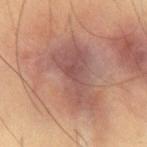{
  "biopsy_status": "not biopsied; imaged during a skin examination",
  "site": "abdomen",
  "image": {
    "source": "total-body photography crop",
    "field_of_view_mm": 15
  },
  "patient": {
    "sex": "male",
    "age_approx": 55
  }
}